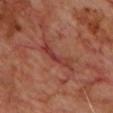– biopsy status: imaged on a skin check; not biopsied
– subject: male, aged approximately 70
– illumination: cross-polarized
– lesion diameter: about 4.5 mm
– body site: the chest
– imaging modality: total-body-photography crop, ~15 mm field of view